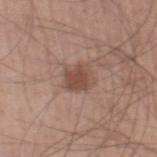patient:
  sex: male
  age_approx: 45
automated_metrics:
  cielab_L: 48
  cielab_a: 20
  cielab_b: 26
  vs_skin_darker_L: 10.0
  lesion_detection_confidence_0_100: 100
image:
  source: total-body photography crop
  field_of_view_mm: 15
site: right thigh
lighting: white-light
lesion_size:
  long_diameter_mm_approx: 3.0